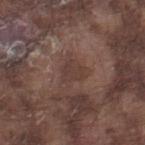| feature | finding |
|---|---|
| automated lesion analysis | a border-irregularity rating of about 3/10, a within-lesion color-variation index near 1.5/10, and radial color variation of about 0.5; a classifier nevus-likeness of about 0/100 and a lesion-detection confidence of about 100/100 |
| subject | male, aged approximately 75 |
| lesion size | ~2.5 mm (longest diameter) |
| acquisition | total-body-photography crop, ~15 mm field of view |
| site | the right lower leg |
| lighting | white-light |